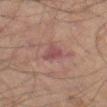notes: catalogued during a skin exam; not biopsied
imaging modality: ~15 mm crop, total-body skin-cancer survey
body site: the left thigh
image-analysis metrics: lesion-presence confidence of about 100/100
lesion diameter: about 3 mm
tile lighting: cross-polarized illumination
subject: male, about 70 years old